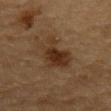notes: catalogued during a skin exam; not biopsied
size: about 6 mm
image source: ~15 mm crop, total-body skin-cancer survey
lighting: cross-polarized
anatomic site: the mid back
patient: male, aged approximately 85 or older